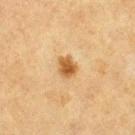Assessment: The lesion was tiled from a total-body skin photograph and was not biopsied. Background: An algorithmic analysis of the crop reported roughly 12 lightness units darker than nearby skin and a lesion-to-skin contrast of about 9.5 (normalized; higher = more distinct). The analysis additionally found border irregularity of about 1.5 on a 0–10 scale, a color-variation rating of about 3/10, and a peripheral color-asymmetry measure near 1. The lesion is on the left thigh. A region of skin cropped from a whole-body photographic capture, roughly 15 mm wide. Longest diameter approximately 2.5 mm. The patient is a female in their mid-50s.